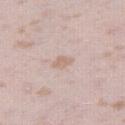The total-body-photography lesion software estimated a lesion color around L≈66 a*≈15 b*≈25 in CIELAB and a normalized border contrast of about 5.5. About 2.5 mm across. A 15 mm close-up extracted from a 3D total-body photography capture. The subject is a female in their mid-20s. The tile uses white-light illumination. The lesion is located on the right thigh.